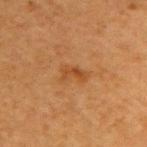biopsy status: total-body-photography surveillance lesion; no biopsy | anatomic site: the right upper arm | lighting: cross-polarized illumination | lesion size: ~2.5 mm (longest diameter) | acquisition: ~15 mm tile from a whole-body skin photo | patient: female, about 40 years old.